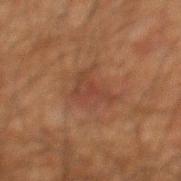{"biopsy_status": "not biopsied; imaged during a skin examination", "patient": {"sex": "male", "age_approx": 60}, "image": {"source": "total-body photography crop", "field_of_view_mm": 15}, "automated_metrics": {"cielab_L": 32, "cielab_a": 18, "cielab_b": 24, "vs_skin_darker_L": 5.0, "vs_skin_contrast_norm": 5.0, "nevus_likeness_0_100": 0}, "lighting": "cross-polarized", "site": "back", "lesion_size": {"long_diameter_mm_approx": 5.0}}